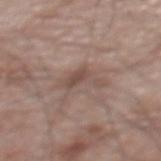workup: no biopsy performed (imaged during a skin exam) | size: ~5 mm (longest diameter) | anatomic site: the upper back | image source: ~15 mm crop, total-body skin-cancer survey | patient: male, aged 68 to 72.